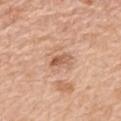{
  "biopsy_status": "not biopsied; imaged during a skin examination",
  "site": "mid back",
  "automated_metrics": {
    "border_irregularity_0_10": 2.5,
    "color_variation_0_10": 2.5
  },
  "lighting": "white-light",
  "image": {
    "source": "total-body photography crop",
    "field_of_view_mm": 15
  },
  "patient": {
    "sex": "female",
    "age_approx": 65
  }
}